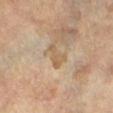Part of a total-body skin-imaging series; this lesion was reviewed on a skin check and was not flagged for biopsy. The patient is a female aged 68 to 72. Automated tile analysis of the lesion measured an area of roughly 4 mm² and a shape eccentricity near 0.9. The software also gave a nevus-likeness score of about 0/100 and a lesion-detection confidence of about 100/100. The lesion is on the right lower leg. About 3.5 mm across. This image is a 15 mm lesion crop taken from a total-body photograph.